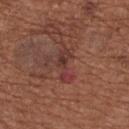Q: Was this lesion biopsied?
A: imaged on a skin check; not biopsied
Q: What kind of image is this?
A: total-body-photography crop, ~15 mm field of view
Q: Lesion location?
A: the upper back
Q: What lighting was used for the tile?
A: white-light illumination
Q: Lesion size?
A: ~4 mm (longest diameter)
Q: Who is the patient?
A: female, in their mid-60s